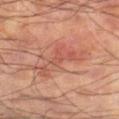  biopsy_status: not biopsied; imaged during a skin examination
  image:
    source: total-body photography crop
    field_of_view_mm: 15
  site: leg
  lighting: cross-polarized
  patient:
    sex: male
    age_approx: 60